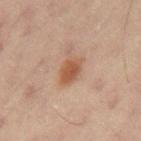Q: Was this lesion biopsied?
A: imaged on a skin check; not biopsied
Q: Lesion size?
A: ~3.5 mm (longest diameter)
Q: What kind of image is this?
A: ~15 mm crop, total-body skin-cancer survey
Q: Patient demographics?
A: male, roughly 60 years of age
Q: What is the anatomic site?
A: the mid back
Q: Automated lesion metrics?
A: two-axis asymmetry of about 0.15; a border-irregularity rating of about 2/10, a within-lesion color-variation index near 2.5/10, and peripheral color asymmetry of about 0.5